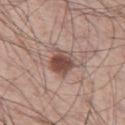<lesion>
  <biopsy_status>not biopsied; imaged during a skin examination</biopsy_status>
  <image>
    <source>total-body photography crop</source>
    <field_of_view_mm>15</field_of_view_mm>
  </image>
  <automated_metrics>
    <cielab_L>48</cielab_L>
    <cielab_a>20</cielab_a>
    <cielab_b>24</cielab_b>
    <border_irregularity_0_10>2.0</border_irregularity_0_10>
    <color_variation_0_10>4.0</color_variation_0_10>
    <peripheral_color_asymmetry>1.0</peripheral_color_asymmetry>
    <nevus_likeness_0_100>95</nevus_likeness_0_100>
  </automated_metrics>
  <site>left thigh</site>
  <lesion_size>
    <long_diameter_mm_approx>3.0</long_diameter_mm_approx>
  </lesion_size>
  <patient>
    <sex>male</sex>
    <age_approx>60</age_approx>
  </patient>
</lesion>